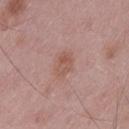notes: imaged on a skin check; not biopsied.